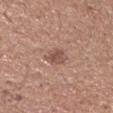Part of a total-body skin-imaging series; this lesion was reviewed on a skin check and was not flagged for biopsy.
A male subject, aged around 65.
Imaged with white-light lighting.
A 15 mm close-up tile from a total-body photography series done for melanoma screening.
Longest diameter approximately 2.5 mm.
Located on the head or neck.
The total-body-photography lesion software estimated a lesion area of about 5 mm² and a shape-asymmetry score of about 0.2 (0 = symmetric). And it measured an average lesion color of about L≈52 a*≈20 b*≈25 (CIELAB), a lesion–skin lightness drop of about 9, and a normalized border contrast of about 6.5. The software also gave a border-irregularity index near 2/10. The software also gave a lesion-detection confidence of about 100/100.